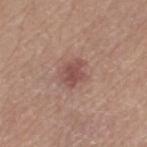Impression:
The lesion was photographed on a routine skin check and not biopsied; there is no pathology result.
Image and clinical context:
About 3 mm across. The tile uses white-light illumination. A female subject aged around 75. A region of skin cropped from a whole-body photographic capture, roughly 15 mm wide. Automated tile analysis of the lesion measured an average lesion color of about L≈49 a*≈22 b*≈23 (CIELAB) and a normalized border contrast of about 7. And it measured a border-irregularity index near 2.5/10, internal color variation of about 2 on a 0–10 scale, and a peripheral color-asymmetry measure near 0.5. And it measured an automated nevus-likeness rating near 65 out of 100 and lesion-presence confidence of about 100/100. Located on the left thigh.